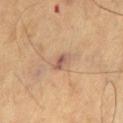Captured during whole-body skin photography for melanoma surveillance; the lesion was not biopsied. Imaged with cross-polarized lighting. A roughly 15 mm field-of-view crop from a total-body skin photograph. Approximately 3 mm at its widest. A male subject, in their mid-60s. Located on the left thigh. Automated image analysis of the tile measured a footprint of about 3 mm², an outline eccentricity of about 0.85 (0 = round, 1 = elongated), and a symmetry-axis asymmetry near 0.3. The software also gave a classifier nevus-likeness of about 0/100.